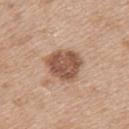acquisition — 15 mm crop, total-body photography; subject — female, roughly 45 years of age; location — the upper back; histopathologic diagnosis — a dysplastic (Clark) nevus.